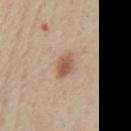{
  "biopsy_status": "not biopsied; imaged during a skin examination",
  "patient": {
    "sex": "male",
    "age_approx": 60
  },
  "automated_metrics": {
    "cielab_L": 57,
    "cielab_a": 19,
    "cielab_b": 30,
    "vs_skin_darker_L": 11.0,
    "vs_skin_contrast_norm": 8.0,
    "color_variation_0_10": 4.5
  },
  "lighting": "white-light",
  "image": {
    "source": "total-body photography crop",
    "field_of_view_mm": 15
  },
  "site": "chest"
}